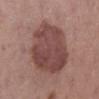Q: Was a biopsy performed?
A: imaged on a skin check; not biopsied
Q: Where on the body is the lesion?
A: the right lower leg
Q: Automated lesion metrics?
A: a mean CIELAB color near L≈45 a*≈22 b*≈21, roughly 12 lightness units darker than nearby skin, and a normalized border contrast of about 9; a border-irregularity index near 2/10, a within-lesion color-variation index near 4/10, and peripheral color asymmetry of about 1.5
Q: What kind of image is this?
A: ~15 mm crop, total-body skin-cancer survey
Q: Who is the patient?
A: female, roughly 55 years of age
Q: How was the tile lit?
A: white-light